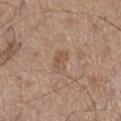No biopsy was performed on this lesion — it was imaged during a full skin examination and was not determined to be concerning. The lesion is located on the leg. A 15 mm crop from a total-body photograph taken for skin-cancer surveillance. Captured under white-light illumination. A male patient about 60 years old. The recorded lesion diameter is about 2.5 mm.